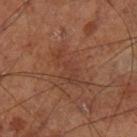Notes:
- notes: catalogued during a skin exam; not biopsied
- location: the left lower leg
- illumination: cross-polarized illumination
- patient: male, in their 70s
- lesion diameter: ≈5 mm
- acquisition: ~15 mm crop, total-body skin-cancer survey